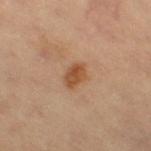Q: Was this lesion biopsied?
A: no biopsy performed (imaged during a skin exam)
Q: How was this image acquired?
A: total-body-photography crop, ~15 mm field of view
Q: Lesion location?
A: the right thigh
Q: Illumination type?
A: cross-polarized
Q: Who is the patient?
A: female, approximately 70 years of age
Q: What is the lesion's diameter?
A: about 3 mm
Q: Automated lesion metrics?
A: a border-irregularity index near 2/10, a within-lesion color-variation index near 3.5/10, and radial color variation of about 1.5; an automated nevus-likeness rating near 85 out of 100